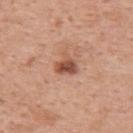A female patient aged approximately 50. A roughly 15 mm field-of-view crop from a total-body skin photograph. Located on the upper back. The tile uses white-light illumination. The recorded lesion diameter is about 2.5 mm.